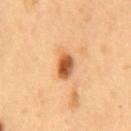Impression:
No biopsy was performed on this lesion — it was imaged during a full skin examination and was not determined to be concerning.
Background:
Imaged with cross-polarized lighting. The lesion-visualizer software estimated a lesion area of about 6 mm² and an outline eccentricity of about 0.7 (0 = round, 1 = elongated). The analysis additionally found an average lesion color of about L≈58 a*≈27 b*≈42 (CIELAB), a lesion–skin lightness drop of about 17, and a normalized lesion–skin contrast near 10.5. And it measured lesion-presence confidence of about 100/100. A 15 mm close-up extracted from a 3D total-body photography capture. Measured at roughly 3 mm in maximum diameter. Located on the mid back. A male patient, aged approximately 50.